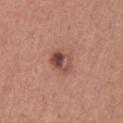| key | value |
|---|---|
| follow-up | catalogued during a skin exam; not biopsied |
| body site | the chest |
| automated lesion analysis | an area of roughly 6 mm² and an eccentricity of roughly 0.6; a border-irregularity index near 2/10 and a within-lesion color-variation index near 10/10 |
| imaging modality | ~15 mm crop, total-body skin-cancer survey |
| patient | male, approximately 45 years of age |